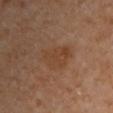Clinical impression: The lesion was tiled from a total-body skin photograph and was not biopsied. Image and clinical context: A 15 mm crop from a total-body photograph taken for skin-cancer surveillance. A female patient, aged 48 to 52. The lesion-visualizer software estimated a lesion area of about 9.5 mm² and an outline eccentricity of about 0.6 (0 = round, 1 = elongated). The analysis additionally found a classifier nevus-likeness of about 5/100 and a detector confidence of about 100 out of 100 that the crop contains a lesion. The lesion's longest dimension is about 4 mm. The lesion is located on the right upper arm.